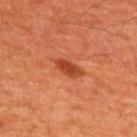| feature | finding |
|---|---|
| TBP lesion metrics | a footprint of about 4.5 mm², an outline eccentricity of about 0.85 (0 = round, 1 = elongated), and two-axis asymmetry of about 0.25; a mean CIELAB color near L≈35 a*≈27 b*≈32, about 9 CIELAB-L* units darker than the surrounding skin, and a lesion-to-skin contrast of about 8 (normalized; higher = more distinct); a classifier nevus-likeness of about 95/100 and a lesion-detection confidence of about 100/100 |
| image source | total-body-photography crop, ~15 mm field of view |
| patient | male, aged 58 to 62 |
| lesion diameter | ~3.5 mm (longest diameter) |
| illumination | cross-polarized |
| site | the back |